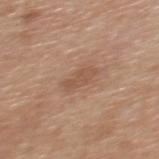This lesion was catalogued during total-body skin photography and was not selected for biopsy.
Imaged with white-light lighting.
A male patient, aged around 30.
A roughly 15 mm field-of-view crop from a total-body skin photograph.
Located on the upper back.
Automated image analysis of the tile measured a mean CIELAB color near L≈53 a*≈20 b*≈30 and a lesion-to-skin contrast of about 5.5 (normalized; higher = more distinct). The analysis additionally found border irregularity of about 4 on a 0–10 scale, a color-variation rating of about 0.5/10, and radial color variation of about 0.
Approximately 3.5 mm at its widest.